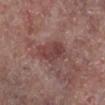{
  "biopsy_status": "not biopsied; imaged during a skin examination",
  "lighting": "white-light",
  "automated_metrics": {
    "area_mm2_approx": 10.0,
    "eccentricity": 0.75,
    "shape_asymmetry": 0.25
  },
  "site": "left lower leg",
  "patient": {
    "sex": "male",
    "age_approx": 65
  },
  "image": {
    "source": "total-body photography crop",
    "field_of_view_mm": 15
  }
}